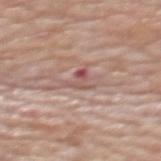{
  "biopsy_status": "not biopsied; imaged during a skin examination",
  "automated_metrics": {
    "area_mm2_approx": 3.5,
    "eccentricity": 0.6,
    "shape_asymmetry": 0.55,
    "cielab_L": 54,
    "cielab_a": 22,
    "cielab_b": 22,
    "vs_skin_darker_L": 10.0,
    "border_irregularity_0_10": 7.0,
    "peripheral_color_asymmetry": 1.5,
    "nevus_likeness_0_100": 0
  },
  "patient": {
    "sex": "female",
    "age_approx": 65
  },
  "image": {
    "source": "total-body photography crop",
    "field_of_view_mm": 15
  },
  "site": "upper back"
}